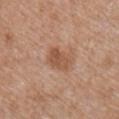The lesion was tiled from a total-body skin photograph and was not biopsied. A male patient approximately 65 years of age. Imaged with white-light lighting. The lesion's longest dimension is about 3.5 mm. A roughly 15 mm field-of-view crop from a total-body skin photograph. The total-body-photography lesion software estimated two-axis asymmetry of about 0.2. The software also gave an average lesion color of about L≈53 a*≈21 b*≈32 (CIELAB), about 9 CIELAB-L* units darker than the surrounding skin, and a lesion-to-skin contrast of about 6.5 (normalized; higher = more distinct). The lesion is on the right upper arm.